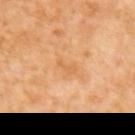workup = total-body-photography surveillance lesion; no biopsy
acquisition = ~15 mm crop, total-body skin-cancer survey
patient = female, aged approximately 55
anatomic site = the upper back
tile lighting = cross-polarized illumination
size = about 2.5 mm